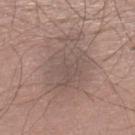| feature | finding |
|---|---|
| notes | imaged on a skin check; not biopsied |
| location | the left lower leg |
| lesion diameter | ≈9 mm |
| subject | male, about 40 years old |
| tile lighting | white-light illumination |
| image source | 15 mm crop, total-body photography |